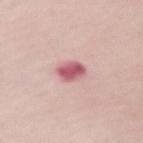{
  "biopsy_status": "not biopsied; imaged during a skin examination",
  "patient": {
    "sex": "female",
    "age_approx": 65
  },
  "image": {
    "source": "total-body photography crop",
    "field_of_view_mm": 15
  },
  "site": "left forearm"
}